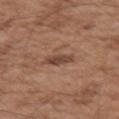No biopsy was performed on this lesion — it was imaged during a full skin examination and was not determined to be concerning. On the left upper arm. A roughly 15 mm field-of-view crop from a total-body skin photograph. Captured under white-light illumination. Approximately 3.5 mm at its widest. The subject is a male about 55 years old.